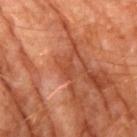Impression: This lesion was catalogued during total-body skin photography and was not selected for biopsy. Acquisition and patient details: This is a cross-polarized tile. The lesion is on the right upper arm. A roughly 15 mm field-of-view crop from a total-body skin photograph. Approximately 2.5 mm at its widest. A male patient aged 58 to 62.